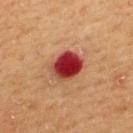No biopsy was performed on this lesion — it was imaged during a full skin examination and was not determined to be concerning. This is a cross-polarized tile. A male subject, aged 58–62. Measured at roughly 4 mm in maximum diameter. Located on the back. A lesion tile, about 15 mm wide, cut from a 3D total-body photograph.